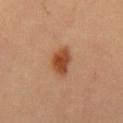Q: Was a biopsy performed?
A: no biopsy performed (imaged during a skin exam)